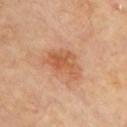Clinical impression: Part of a total-body skin-imaging series; this lesion was reviewed on a skin check and was not flagged for biopsy. Background: A region of skin cropped from a whole-body photographic capture, roughly 15 mm wide. Captured under cross-polarized illumination. The recorded lesion diameter is about 5 mm. Located on the chest.